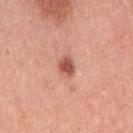{"biopsy_status": "not biopsied; imaged during a skin examination", "patient": {"sex": "female", "age_approx": 40}, "lighting": "white-light", "image": {"source": "total-body photography crop", "field_of_view_mm": 15}, "site": "right upper arm"}